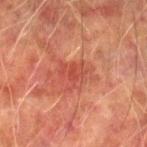| field | value |
|---|---|
| workup | total-body-photography surveillance lesion; no biopsy |